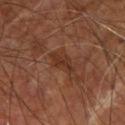Impression: The lesion was tiled from a total-body skin photograph and was not biopsied. Clinical summary: The total-body-photography lesion software estimated a lesion–skin lightness drop of about 7 and a normalized lesion–skin contrast near 6.5. This is a cross-polarized tile. The lesion is on the right upper arm. A subject aged 63 to 67. About 3.5 mm across. This image is a 15 mm lesion crop taken from a total-body photograph.